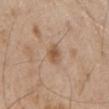Recorded during total-body skin imaging; not selected for excision or biopsy. A 15 mm crop from a total-body photograph taken for skin-cancer surveillance. On the chest. A male patient aged 63–67. The lesion-visualizer software estimated a lesion color around L≈57 a*≈17 b*≈30 in CIELAB and about 7 CIELAB-L* units darker than the surrounding skin. About 3.5 mm across.